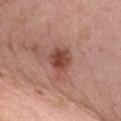Captured during whole-body skin photography for melanoma surveillance; the lesion was not biopsied.
On the chest.
A female patient, approximately 40 years of age.
A lesion tile, about 15 mm wide, cut from a 3D total-body photograph.
About 3.5 mm across.